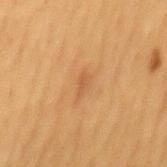notes: imaged on a skin check; not biopsied | subject: male, aged approximately 55 | size: ~2.5 mm (longest diameter) | illumination: cross-polarized illumination | image source: total-body-photography crop, ~15 mm field of view | image-analysis metrics: an outline eccentricity of about 0.9 (0 = round, 1 = elongated) and a symmetry-axis asymmetry near 0.35; a nevus-likeness score of about 0/100 and a detector confidence of about 100 out of 100 that the crop contains a lesion | site: the mid back.